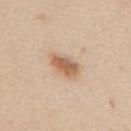follow-up — no biopsy performed (imaged during a skin exam); imaging modality — 15 mm crop, total-body photography; subject — female, aged around 45; body site — the upper back.